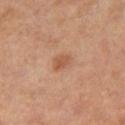Q: Is there a histopathology result?
A: no biopsy performed (imaged during a skin exam)
Q: Lesion size?
A: ~2.5 mm (longest diameter)
Q: What are the patient's age and sex?
A: male, aged 53 to 57
Q: Illumination type?
A: cross-polarized
Q: Lesion location?
A: the right thigh
Q: What is the imaging modality?
A: ~15 mm crop, total-body skin-cancer survey